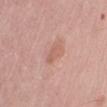image:
  source: total-body photography crop
  field_of_view_mm: 15
site: left upper arm
patient:
  sex: male
  age_approx: 65
lesion_size:
  long_diameter_mm_approx: 2.5
automated_metrics:
  border_irregularity_0_10: 3.5
  color_variation_0_10: 0.5
  peripheral_color_asymmetry: 0.0
  nevus_likeness_0_100: 0
  lesion_detection_confidence_0_100: 100
lighting: white-light